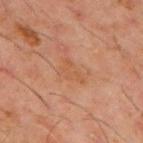Q: Was this lesion biopsied?
A: catalogued during a skin exam; not biopsied
Q: What kind of image is this?
A: ~15 mm crop, total-body skin-cancer survey
Q: What is the lesion's diameter?
A: ≈3.5 mm
Q: Illumination type?
A: cross-polarized
Q: Who is the patient?
A: male, approximately 60 years of age
Q: Where on the body is the lesion?
A: the mid back